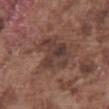The lesion was photographed on a routine skin check and not biopsied; there is no pathology result. A region of skin cropped from a whole-body photographic capture, roughly 15 mm wide. The patient is a male roughly 75 years of age. Automated image analysis of the tile measured a mean CIELAB color near L≈38 a*≈17 b*≈21, about 8 CIELAB-L* units darker than the surrounding skin, and a normalized lesion–skin contrast near 7.5. The lesion is on the front of the torso. Captured under white-light illumination.